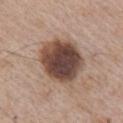biopsy status: total-body-photography surveillance lesion; no biopsy | site: the arm | image: total-body-photography crop, ~15 mm field of view | patient: male, aged around 65.